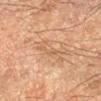follow-up: imaged on a skin check; not biopsied
location: the arm
subject: male, aged around 65
illumination: cross-polarized
automated lesion analysis: a shape eccentricity near 0.95 and a shape-asymmetry score of about 0.5 (0 = symmetric); a lesion color around L≈52 a*≈17 b*≈31 in CIELAB, a lesion–skin lightness drop of about 6, and a lesion-to-skin contrast of about 4.5 (normalized; higher = more distinct); a border-irregularity index near 7/10; a classifier nevus-likeness of about 0/100 and a lesion-detection confidence of about 50/100
acquisition: 15 mm crop, total-body photography
size: ~5.5 mm (longest diameter)